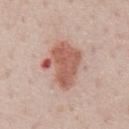This lesion was catalogued during total-body skin photography and was not selected for biopsy.
Cropped from a total-body skin-imaging series; the visible field is about 15 mm.
The lesion is on the abdomen.
This is a white-light tile.
Longest diameter approximately 5.5 mm.
The total-body-photography lesion software estimated an automated nevus-likeness rating near 5 out of 100 and lesion-presence confidence of about 100/100.
A male subject, roughly 60 years of age.